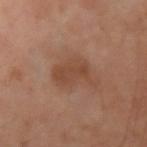{
  "biopsy_status": "not biopsied; imaged during a skin examination",
  "automated_metrics": {
    "area_mm2_approx": 11.0,
    "eccentricity": 0.75,
    "shape_asymmetry": 0.35,
    "border_irregularity_0_10": 4.0,
    "color_variation_0_10": 2.0,
    "peripheral_color_asymmetry": 0.5
  },
  "site": "left arm",
  "image": {
    "source": "total-body photography crop",
    "field_of_view_mm": 15
  },
  "lesion_size": {
    "long_diameter_mm_approx": 4.5
  },
  "lighting": "cross-polarized",
  "patient": {
    "sex": "male",
    "age_approx": 50
  }
}